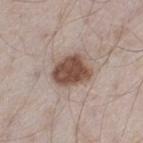The lesion was photographed on a routine skin check and not biopsied; there is no pathology result. Cropped from a total-body skin-imaging series; the visible field is about 15 mm. Located on the left thigh. The patient is a male aged around 45.